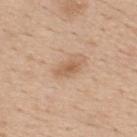Assessment: This lesion was catalogued during total-body skin photography and was not selected for biopsy. Image and clinical context: The patient is a male approximately 60 years of age. On the upper back. Approximately 3 mm at its widest. A close-up tile cropped from a whole-body skin photograph, about 15 mm across. This is a white-light tile.